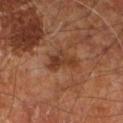Notes:
– notes: total-body-photography surveillance lesion; no biopsy
– patient: male, roughly 60 years of age
– body site: the right leg
– image source: ~15 mm tile from a whole-body skin photo
– lighting: cross-polarized
– size: ≈4 mm